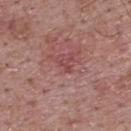The lesion was tiled from a total-body skin photograph and was not biopsied. A close-up tile cropped from a whole-body skin photograph, about 15 mm across. This is a white-light tile. The lesion is located on the back. Longest diameter approximately 1.5 mm. A male subject aged approximately 70.